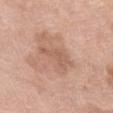Q: Who is the patient?
A: female, in their mid-70s
Q: What did automated image analysis measure?
A: a footprint of about 6 mm², an eccentricity of roughly 0.9, and a shape-asymmetry score of about 0.55 (0 = symmetric); a detector confidence of about 100 out of 100 that the crop contains a lesion
Q: What lighting was used for the tile?
A: white-light illumination
Q: What is the imaging modality?
A: 15 mm crop, total-body photography
Q: Where on the body is the lesion?
A: the front of the torso